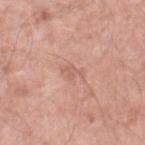biopsy status — no biopsy performed (imaged during a skin exam)
size — about 2.5 mm
body site — the right lower leg
subject — male, aged 48 to 52
image source — ~15 mm crop, total-body skin-cancer survey
lighting — white-light